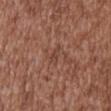<record>
  <biopsy_status>not biopsied; imaged during a skin examination</biopsy_status>
  <lesion_size>
    <long_diameter_mm_approx>2.5</long_diameter_mm_approx>
  </lesion_size>
  <image>
    <source>total-body photography crop</source>
    <field_of_view_mm>15</field_of_view_mm>
  </image>
  <automated_metrics>
    <vs_skin_contrast_norm>5.0</vs_skin_contrast_norm>
    <color_variation_0_10>2.0</color_variation_0_10>
    <peripheral_color_asymmetry>0.5</peripheral_color_asymmetry>
    <nevus_likeness_0_100>0</nevus_likeness_0_100>
    <lesion_detection_confidence_0_100>100</lesion_detection_confidence_0_100>
  </automated_metrics>
  <patient>
    <sex>male</sex>
    <age_approx>45</age_approx>
  </patient>
  <site>abdomen</site>
</record>